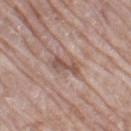Q: Was a biopsy performed?
A: no biopsy performed (imaged during a skin exam)
Q: How large is the lesion?
A: ~4 mm (longest diameter)
Q: How was this image acquired?
A: ~15 mm tile from a whole-body skin photo
Q: Patient demographics?
A: female, in their mid- to late 70s
Q: Where on the body is the lesion?
A: the left thigh
Q: What did automated image analysis measure?
A: an average lesion color of about L≈53 a*≈18 b*≈24 (CIELAB), about 9 CIELAB-L* units darker than the surrounding skin, and a normalized border contrast of about 6.5; border irregularity of about 4.5 on a 0–10 scale, internal color variation of about 3 on a 0–10 scale, and peripheral color asymmetry of about 1; a nevus-likeness score of about 0/100 and a lesion-detection confidence of about 60/100